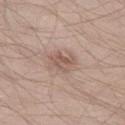<record>
<biopsy_status>not biopsied; imaged during a skin examination</biopsy_status>
<site>left thigh</site>
<patient>
  <sex>male</sex>
  <age_approx>35</age_approx>
</patient>
<image>
  <source>total-body photography crop</source>
  <field_of_view_mm>15</field_of_view_mm>
</image>
</record>